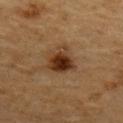Impression:
Imaged during a routine full-body skin examination; the lesion was not biopsied and no histopathology is available.
Image and clinical context:
This image is a 15 mm lesion crop taken from a total-body photograph. This is a cross-polarized tile. The lesion is located on the upper back. A male subject, aged approximately 85 or older.